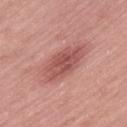| key | value |
|---|---|
| biopsy status | total-body-photography surveillance lesion; no biopsy |
| lesion diameter | ≈6 mm |
| image-analysis metrics | a shape eccentricity near 0.9 and two-axis asymmetry of about 0.15; a lesion color around L≈53 a*≈27 b*≈25 in CIELAB, roughly 11 lightness units darker than nearby skin, and a lesion-to-skin contrast of about 7.5 (normalized; higher = more distinct); a border-irregularity rating of about 2.5/10, a within-lesion color-variation index near 3.5/10, and a peripheral color-asymmetry measure near 1 |
| subject | male, aged 63 to 67 |
| imaging modality | 15 mm crop, total-body photography |
| location | the leg |
| tile lighting | white-light |